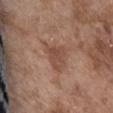biopsy status: total-body-photography surveillance lesion; no biopsy
anatomic site: the front of the torso
subject: male, in their mid- to late 70s
imaging modality: total-body-photography crop, ~15 mm field of view
TBP lesion metrics: roughly 8 lightness units darker than nearby skin and a lesion-to-skin contrast of about 6.5 (normalized; higher = more distinct); a border-irregularity index near 4.5/10, a color-variation rating of about 2.5/10, and radial color variation of about 1
tile lighting: white-light illumination